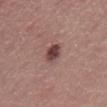No biopsy was performed on this lesion — it was imaged during a full skin examination and was not determined to be concerning.
The subject is a male roughly 40 years of age.
On the front of the torso.
A 15 mm close-up extracted from a 3D total-body photography capture.